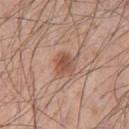Clinical impression:
Part of a total-body skin-imaging series; this lesion was reviewed on a skin check and was not flagged for biopsy.
Context:
This is a white-light tile. A region of skin cropped from a whole-body photographic capture, roughly 15 mm wide. A male subject aged approximately 55. From the front of the torso. Approximately 3 mm at its widest. Automated image analysis of the tile measured a lesion area of about 6 mm² and two-axis asymmetry of about 0.2. The analysis additionally found a lesion-to-skin contrast of about 7.5 (normalized; higher = more distinct). And it measured a border-irregularity index near 2/10, internal color variation of about 4.5 on a 0–10 scale, and peripheral color asymmetry of about 1.5.